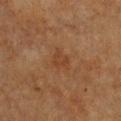This lesion was catalogued during total-body skin photography and was not selected for biopsy. The lesion is located on the front of the torso. A 15 mm close-up tile from a total-body photography series done for melanoma screening. Approximately 2.5 mm at its widest. Imaged with cross-polarized lighting. The subject is a female in their mid- to late 50s.